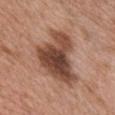workup: total-body-photography surveillance lesion; no biopsy
subject: male, aged around 50
acquisition: total-body-photography crop, ~15 mm field of view
location: the front of the torso
illumination: white-light